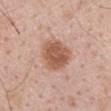| field | value |
|---|---|
| workup | imaged on a skin check; not biopsied |
| subject | male, aged around 55 |
| illumination | white-light illumination |
| image source | ~15 mm tile from a whole-body skin photo |
| body site | the back |